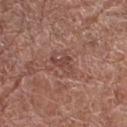- notes — no biopsy performed (imaged during a skin exam)
- image source — ~15 mm tile from a whole-body skin photo
- location — the left lower leg
- subject — female, roughly 80 years of age
- lesion diameter — ~3 mm (longest diameter)
- illumination — white-light
- TBP lesion metrics — an area of roughly 4 mm², an outline eccentricity of about 0.8 (0 = round, 1 = elongated), and a shape-asymmetry score of about 0.5 (0 = symmetric); border irregularity of about 5 on a 0–10 scale, internal color variation of about 1.5 on a 0–10 scale, and a peripheral color-asymmetry measure near 0.5